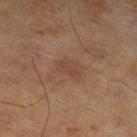Impression:
No biopsy was performed on this lesion — it was imaged during a full skin examination and was not determined to be concerning.
Acquisition and patient details:
The total-body-photography lesion software estimated an average lesion color of about L≈42 a*≈19 b*≈27 (CIELAB) and a lesion-to-skin contrast of about 4.5 (normalized; higher = more distinct). The software also gave a border-irregularity index near 3/10. The analysis additionally found a nevus-likeness score of about 0/100 and a lesion-detection confidence of about 100/100. The lesion's longest dimension is about 3 mm. A lesion tile, about 15 mm wide, cut from a 3D total-body photograph. A male subject, aged 63 to 67.